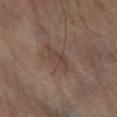The lesion was photographed on a routine skin check and not biopsied; there is no pathology result. Measured at roughly 4.5 mm in maximum diameter. A 15 mm crop from a total-body photograph taken for skin-cancer surveillance. Imaged with cross-polarized lighting. On the right lower leg. The total-body-photography lesion software estimated a mean CIELAB color near L≈41 a*≈16 b*≈21, a lesion–skin lightness drop of about 6, and a normalized border contrast of about 5. A subject about 55 years old.